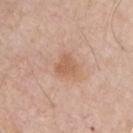Imaged during a routine full-body skin examination; the lesion was not biopsied and no histopathology is available. The lesion is located on the chest. The subject is a male in their mid-60s. Measured at roughly 2.5 mm in maximum diameter. This image is a 15 mm lesion crop taken from a total-body photograph. Imaged with white-light lighting. The total-body-photography lesion software estimated an area of roughly 5 mm², a shape eccentricity near 0.4, and a shape-asymmetry score of about 0.25 (0 = symmetric). The analysis additionally found a lesion color around L≈59 a*≈21 b*≈33 in CIELAB, about 8 CIELAB-L* units darker than the surrounding skin, and a lesion-to-skin contrast of about 6.5 (normalized; higher = more distinct).